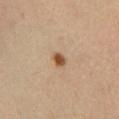Findings:
– site · the leg
– tile lighting · cross-polarized illumination
– acquisition · ~15 mm tile from a whole-body skin photo
– subject · male, aged 38–42